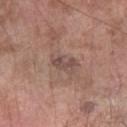No biopsy was performed on this lesion — it was imaged during a full skin examination and was not determined to be concerning.
A region of skin cropped from a whole-body photographic capture, roughly 15 mm wide.
A male subject aged approximately 60.
The recorded lesion diameter is about 3 mm.
The lesion is located on the right forearm.
This is a white-light tile.